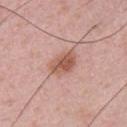Q: Was this lesion biopsied?
A: no biopsy performed (imaged during a skin exam)
Q: What is the lesion's diameter?
A: ~3.5 mm (longest diameter)
Q: What did automated image analysis measure?
A: an outline eccentricity of about 0.75 (0 = round, 1 = elongated) and a symmetry-axis asymmetry near 0.3; a mean CIELAB color near L≈56 a*≈23 b*≈28 and about 11 CIELAB-L* units darker than the surrounding skin; border irregularity of about 2.5 on a 0–10 scale and a within-lesion color-variation index near 4.5/10; a detector confidence of about 100 out of 100 that the crop contains a lesion
Q: What is the anatomic site?
A: the front of the torso
Q: How was the tile lit?
A: white-light illumination
Q: How was this image acquired?
A: ~15 mm crop, total-body skin-cancer survey
Q: Patient demographics?
A: male, in their 50s